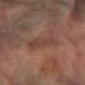Captured during whole-body skin photography for melanoma surveillance; the lesion was not biopsied. On the left forearm. A close-up tile cropped from a whole-body skin photograph, about 15 mm across. A male subject roughly 85 years of age or older.